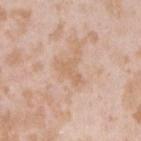Assessment:
Captured during whole-body skin photography for melanoma surveillance; the lesion was not biopsied.
Clinical summary:
A female patient in their mid- to late 20s. The lesion is on the arm. A close-up tile cropped from a whole-body skin photograph, about 15 mm across.